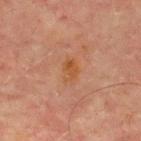Clinical impression: The lesion was tiled from a total-body skin photograph and was not biopsied. Background: On the chest. The lesion-visualizer software estimated an area of roughly 5 mm². The software also gave an average lesion color of about L≈43 a*≈20 b*≈32 (CIELAB), about 5 CIELAB-L* units darker than the surrounding skin, and a normalized border contrast of about 6.5. It also reported a lesion-detection confidence of about 100/100. A region of skin cropped from a whole-body photographic capture, roughly 15 mm wide. The subject is a male in their 70s. This is a cross-polarized tile.